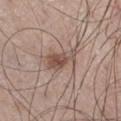This lesion was catalogued during total-body skin photography and was not selected for biopsy. Longest diameter approximately 4 mm. An algorithmic analysis of the crop reported a lesion color around L≈50 a*≈17 b*≈24 in CIELAB, roughly 11 lightness units darker than nearby skin, and a normalized border contrast of about 8. And it measured a lesion-detection confidence of about 100/100. A male subject, approximately 60 years of age. From the right thigh. Captured under white-light illumination. Cropped from a total-body skin-imaging series; the visible field is about 15 mm.